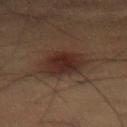Assessment: Imaged during a routine full-body skin examination; the lesion was not biopsied and no histopathology is available. Clinical summary: Cropped from a total-body skin-imaging series; the visible field is about 15 mm. The lesion is on the abdomen. An algorithmic analysis of the crop reported a shape-asymmetry score of about 0.15 (0 = symmetric). The analysis additionally found an average lesion color of about L≈21 a*≈14 b*≈18 (CIELAB) and a lesion–skin lightness drop of about 7. The analysis additionally found an automated nevus-likeness rating near 90 out of 100. The subject is a male in their 50s.